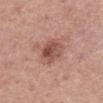Recorded during total-body skin imaging; not selected for excision or biopsy. A male subject, aged around 50. This image is a 15 mm lesion crop taken from a total-body photograph. From the abdomen.